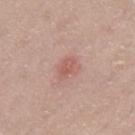This lesion was catalogued during total-body skin photography and was not selected for biopsy.
A region of skin cropped from a whole-body photographic capture, roughly 15 mm wide.
The subject is a female approximately 70 years of age.
The lesion is on the right thigh.
Captured under white-light illumination.